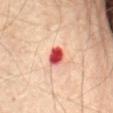Q: How was this image acquired?
A: total-body-photography crop, ~15 mm field of view
Q: What is the anatomic site?
A: the abdomen
Q: Lesion size?
A: about 2.5 mm
Q: Illumination type?
A: cross-polarized
Q: Automated lesion metrics?
A: an area of roughly 4.5 mm², a shape eccentricity near 0.65, and a symmetry-axis asymmetry near 0.25; border irregularity of about 2 on a 0–10 scale and peripheral color asymmetry of about 1.5; a nevus-likeness score of about 0/100
Q: Patient demographics?
A: male, aged 68–72